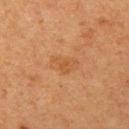Part of a total-body skin-imaging series; this lesion was reviewed on a skin check and was not flagged for biopsy.
About 3.5 mm across.
Automated tile analysis of the lesion measured a mean CIELAB color near L≈45 a*≈21 b*≈34, about 5 CIELAB-L* units darker than the surrounding skin, and a normalized lesion–skin contrast near 4.5. And it measured internal color variation of about 2 on a 0–10 scale and a peripheral color-asymmetry measure near 0.5.
This image is a 15 mm lesion crop taken from a total-body photograph.
From the left upper arm.
A female subject, aged approximately 40.